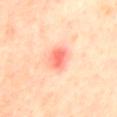Q: Is there a histopathology result?
A: no biopsy performed (imaged during a skin exam)
Q: Lesion size?
A: ~3 mm (longest diameter)
Q: What did automated image analysis measure?
A: an outline eccentricity of about 0.8 (0 = round, 1 = elongated) and two-axis asymmetry of about 0.15; a normalized border contrast of about 7; a nevus-likeness score of about 0/100 and lesion-presence confidence of about 100/100
Q: Who is the patient?
A: female, roughly 40 years of age
Q: Illumination type?
A: cross-polarized
Q: What is the imaging modality?
A: total-body-photography crop, ~15 mm field of view
Q: Where on the body is the lesion?
A: the mid back